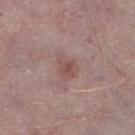Clinical impression:
Part of a total-body skin-imaging series; this lesion was reviewed on a skin check and was not flagged for biopsy.
Clinical summary:
Located on the right lower leg. A male patient aged around 75. Cropped from a total-body skin-imaging series; the visible field is about 15 mm. The lesion-visualizer software estimated a color-variation rating of about 2.5/10 and a peripheral color-asymmetry measure near 1. It also reported a classifier nevus-likeness of about 15/100 and lesion-presence confidence of about 100/100. Measured at roughly 3 mm in maximum diameter. This is a white-light tile.